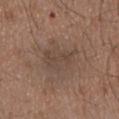follow-up — catalogued during a skin exam; not biopsied | anatomic site — the abdomen | image source — ~15 mm crop, total-body skin-cancer survey | subject — male, aged 73–77.